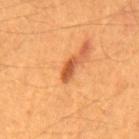Part of a total-body skin-imaging series; this lesion was reviewed on a skin check and was not flagged for biopsy.
A male subject, approximately 60 years of age.
The total-body-photography lesion software estimated a lesion area of about 3 mm² and a symmetry-axis asymmetry near 0.35. The analysis additionally found a mean CIELAB color near L≈50 a*≈30 b*≈42, a lesion–skin lightness drop of about 14, and a normalized lesion–skin contrast near 9.5. And it measured a classifier nevus-likeness of about 95/100.
Captured under cross-polarized illumination.
Cropped from a total-body skin-imaging series; the visible field is about 15 mm.
The lesion is on the back.
The recorded lesion diameter is about 3 mm.